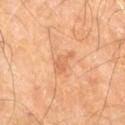follow-up: catalogued during a skin exam; not biopsied | imaging modality: 15 mm crop, total-body photography | patient: male, aged 58–62 | tile lighting: cross-polarized illumination | automated metrics: a color-variation rating of about 1.5/10 and radial color variation of about 0.5 | location: the front of the torso | lesion size: ≈3.5 mm.